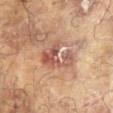{
  "biopsy_status": "not biopsied; imaged during a skin examination",
  "automated_metrics": {
    "vs_skin_darker_L": 9.0,
    "vs_skin_contrast_norm": 7.0,
    "border_irregularity_0_10": 7.0,
    "color_variation_0_10": 6.0,
    "peripheral_color_asymmetry": 2.0,
    "nevus_likeness_0_100": 0,
    "lesion_detection_confidence_0_100": 90
  },
  "patient": {
    "sex": "female",
    "age_approx": 80
  },
  "image": {
    "source": "total-body photography crop",
    "field_of_view_mm": 15
  },
  "lighting": "cross-polarized",
  "lesion_size": {
    "long_diameter_mm_approx": 4.5
  },
  "site": "right arm"
}